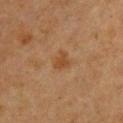Assessment: The lesion was photographed on a routine skin check and not biopsied; there is no pathology result. Context: Approximately 2.5 mm at its widest. The subject is a female roughly 40 years of age. From the chest. A 15 mm crop from a total-body photograph taken for skin-cancer surveillance.